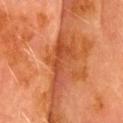– workup: catalogued during a skin exam; not biopsied
– anatomic site: the head or neck
– subject: male, in their 70s
– tile lighting: cross-polarized
– imaging modality: 15 mm crop, total-body photography